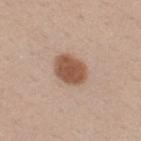workup=catalogued during a skin exam; not biopsied
image=15 mm crop, total-body photography
diameter=≈4 mm
illumination=white-light illumination
anatomic site=the upper back
subject=male, in their 40s